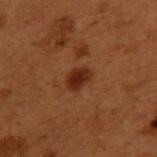This lesion was catalogued during total-body skin photography and was not selected for biopsy.
Cropped from a whole-body photographic skin survey; the tile spans about 15 mm.
The lesion is on the upper back.
A male subject aged 48 to 52.